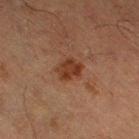The lesion was photographed on a routine skin check and not biopsied; there is no pathology result. Captured under cross-polarized illumination. Measured at roughly 3 mm in maximum diameter. A region of skin cropped from a whole-body photographic capture, roughly 15 mm wide. The lesion is located on the left thigh. A male patient, in their mid-60s. An algorithmic analysis of the crop reported an eccentricity of roughly 0.65 and two-axis asymmetry of about 0.25. The software also gave a mean CIELAB color near L≈29 a*≈19 b*≈26, roughly 8 lightness units darker than nearby skin, and a normalized lesion–skin contrast near 8.5. The software also gave a border-irregularity index near 2.5/10, internal color variation of about 2 on a 0–10 scale, and peripheral color asymmetry of about 1.